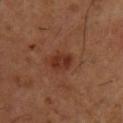No biopsy was performed on this lesion — it was imaged during a full skin examination and was not determined to be concerning.
The patient is a male in their mid-50s.
Cropped from a whole-body photographic skin survey; the tile spans about 15 mm.
Imaged with cross-polarized lighting.
About 3 mm across.
Located on the chest.
An algorithmic analysis of the crop reported a footprint of about 5 mm², a shape eccentricity near 0.75, and a shape-asymmetry score of about 0.25 (0 = symmetric). And it measured a lesion–skin lightness drop of about 8. And it measured a border-irregularity index near 2.5/10, internal color variation of about 3 on a 0–10 scale, and peripheral color asymmetry of about 1. And it measured a classifier nevus-likeness of about 60/100 and a detector confidence of about 100 out of 100 that the crop contains a lesion.